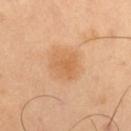{
  "biopsy_status": "not biopsied; imaged during a skin examination",
  "site": "right thigh",
  "lighting": "cross-polarized",
  "patient": {
    "sex": "male",
    "age_approx": 50
  },
  "image": {
    "source": "total-body photography crop",
    "field_of_view_mm": 15
  },
  "automated_metrics": {
    "area_mm2_approx": 9.0,
    "eccentricity": 0.55,
    "shape_asymmetry": 0.15,
    "cielab_L": 62,
    "cielab_a": 22,
    "cielab_b": 39,
    "vs_skin_darker_L": 7.0,
    "vs_skin_contrast_norm": 5.5,
    "border_irregularity_0_10": 1.5,
    "color_variation_0_10": 2.5,
    "peripheral_color_asymmetry": 1.0,
    "lesion_detection_confidence_0_100": 100
  },
  "lesion_size": {
    "long_diameter_mm_approx": 3.5
  }
}